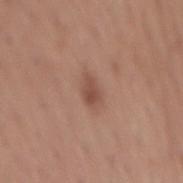{
  "biopsy_status": "not biopsied; imaged during a skin examination",
  "image": {
    "source": "total-body photography crop",
    "field_of_view_mm": 15
  },
  "patient": {
    "sex": "female",
    "age_approx": 65
  },
  "lesion_size": {
    "long_diameter_mm_approx": 3.0
  },
  "site": "mid back",
  "lighting": "white-light",
  "automated_metrics": {
    "area_mm2_approx": 4.5,
    "eccentricity": 0.85,
    "shape_asymmetry": 0.2,
    "border_irregularity_0_10": 2.0,
    "color_variation_0_10": 2.5,
    "peripheral_color_asymmetry": 1.0,
    "nevus_likeness_0_100": 65,
    "lesion_detection_confidence_0_100": 100
  }
}